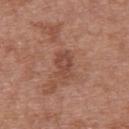A female patient about 40 years old.
A close-up tile cropped from a whole-body skin photograph, about 15 mm across.
On the back.